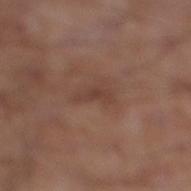Clinical impression: The lesion was photographed on a routine skin check and not biopsied; there is no pathology result. Clinical summary: The patient is a male aged approximately 55. The tile uses white-light illumination. The recorded lesion diameter is about 3.5 mm. The total-body-photography lesion software estimated an area of roughly 3 mm², a shape eccentricity near 0.95, and a symmetry-axis asymmetry near 0.5. And it measured a border-irregularity index near 5.5/10. The analysis additionally found an automated nevus-likeness rating near 0 out of 100 and a detector confidence of about 100 out of 100 that the crop contains a lesion. The lesion is on the leg. A roughly 15 mm field-of-view crop from a total-body skin photograph.